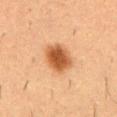Assessment:
Captured during whole-body skin photography for melanoma surveillance; the lesion was not biopsied.